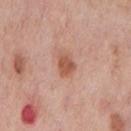A male subject in their mid-70s. Automated tile analysis of the lesion measured an area of roughly 4.5 mm² and a shape eccentricity near 0.65. It also reported border irregularity of about 2 on a 0–10 scale, internal color variation of about 2.5 on a 0–10 scale, and a peripheral color-asymmetry measure near 1. It also reported an automated nevus-likeness rating near 80 out of 100. The lesion is on the front of the torso. Longest diameter approximately 2.5 mm. The tile uses white-light illumination. This image is a 15 mm lesion crop taken from a total-body photograph.